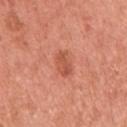Q: Is there a histopathology result?
A: catalogued during a skin exam; not biopsied
Q: How was this image acquired?
A: ~15 mm crop, total-body skin-cancer survey
Q: What did automated image analysis measure?
A: a border-irregularity index near 2.5/10 and a peripheral color-asymmetry measure near 0.5
Q: Patient demographics?
A: female, aged 48 to 52
Q: Lesion location?
A: the right upper arm
Q: What lighting was used for the tile?
A: white-light illumination